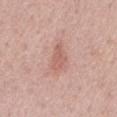The lesion was tiled from a total-body skin photograph and was not biopsied.
The recorded lesion diameter is about 4 mm.
The subject is a male aged 68 to 72.
A 15 mm close-up tile from a total-body photography series done for melanoma screening.
The lesion is on the abdomen.
The tile uses white-light illumination.
Automated image analysis of the tile measured an area of roughly 4.5 mm², an eccentricity of roughly 0.9, and a symmetry-axis asymmetry near 0.3. And it measured a mean CIELAB color near L≈60 a*≈24 b*≈25. And it measured a border-irregularity index near 4/10 and a within-lesion color-variation index near 1/10. The software also gave a classifier nevus-likeness of about 10/100 and a detector confidence of about 100 out of 100 that the crop contains a lesion.